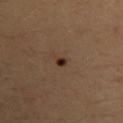biopsy_status: not biopsied; imaged during a skin examination
automated_metrics:
  cielab_L: 29
  cielab_a: 18
  cielab_b: 25
  vs_skin_darker_L: 12.0
site: left upper arm
patient:
  sex: female
  age_approx: 35
image:
  source: total-body photography crop
  field_of_view_mm: 15
lesion_size:
  long_diameter_mm_approx: 1.5
lighting: cross-polarized